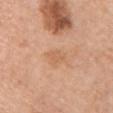Part of a total-body skin-imaging series; this lesion was reviewed on a skin check and was not flagged for biopsy. Located on the left upper arm. A female patient aged around 60. This image is a 15 mm lesion crop taken from a total-body photograph. Longest diameter approximately 2.5 mm.